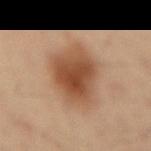Notes:
- biopsy status — imaged on a skin check; not biopsied
- image — 15 mm crop, total-body photography
- patient — male, aged around 35
- site — the back
- automated lesion analysis — an area of roughly 23 mm², a shape eccentricity near 0.65, and a symmetry-axis asymmetry near 0.2; a within-lesion color-variation index near 5/10 and a peripheral color-asymmetry measure near 1.5